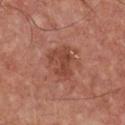Clinical impression: Recorded during total-body skin imaging; not selected for excision or biopsy. Image and clinical context: Longest diameter approximately 4 mm. This image is a 15 mm lesion crop taken from a total-body photograph. Captured under white-light illumination. The total-body-photography lesion software estimated about 9 CIELAB-L* units darker than the surrounding skin. The software also gave a within-lesion color-variation index near 3/10. From the chest. The subject is a male in their mid- to late 60s.